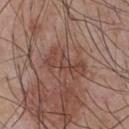Recorded during total-body skin imaging; not selected for excision or biopsy. Automated tile analysis of the lesion measured a lesion area of about 7.5 mm² and a symmetry-axis asymmetry near 0.45. The analysis additionally found a border-irregularity index near 8/10, a color-variation rating of about 2.5/10, and peripheral color asymmetry of about 0.5. This image is a 15 mm lesion crop taken from a total-body photograph. This is a white-light tile. A male patient in their mid-50s. Located on the chest. About 5 mm across.